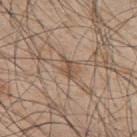biopsy status=catalogued during a skin exam; not biopsied | imaging modality=total-body-photography crop, ~15 mm field of view | anatomic site=the upper back | patient=male, aged 43–47.